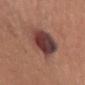notes = total-body-photography surveillance lesion; no biopsy
body site = the right upper arm
subject = female, aged 33 to 37
image = ~15 mm crop, total-body skin-cancer survey
illumination = white-light illumination